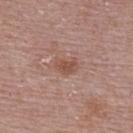notes — imaged on a skin check; not biopsied | patient — female, aged approximately 65 | body site — the back | image — ~15 mm tile from a whole-body skin photo.